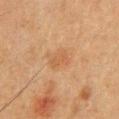Recorded during total-body skin imaging; not selected for excision or biopsy.
A male patient approximately 50 years of age.
The recorded lesion diameter is about 3 mm.
A 15 mm close-up extracted from a 3D total-body photography capture.
The lesion is located on the chest.